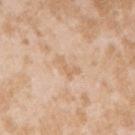The lesion was tiled from a total-body skin photograph and was not biopsied.
About 3.5 mm across.
A female patient, aged 23 to 27.
Captured under white-light illumination.
Located on the arm.
A roughly 15 mm field-of-view crop from a total-body skin photograph.